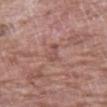This lesion was catalogued during total-body skin photography and was not selected for biopsy.
A female patient about 70 years old.
Located on the left forearm.
This is a white-light tile.
A 15 mm close-up extracted from a 3D total-body photography capture.